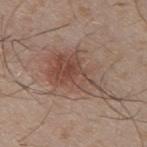Case summary:
- biopsy status · imaged on a skin check; not biopsied
- subject · male, roughly 30 years of age
- diameter · about 8 mm
- imaging modality · ~15 mm crop, total-body skin-cancer survey
- automated metrics · an eccentricity of roughly 0.85 and a shape-asymmetry score of about 0.4 (0 = symmetric)
- body site · the upper back
- illumination · white-light illumination